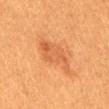workup: catalogued during a skin exam; not biopsied | illumination: cross-polarized | subject: female, roughly 55 years of age | acquisition: ~15 mm crop, total-body skin-cancer survey | lesion size: ≈5 mm | site: the abdomen.